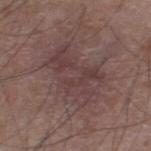<tbp_lesion>
  <image>
    <source>total-body photography crop</source>
    <field_of_view_mm>15</field_of_view_mm>
  </image>
  <patient>
    <sex>male</sex>
    <age_approx>60</age_approx>
  </patient>
  <site>right lower leg</site>
</tbp_lesion>